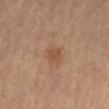• notes: total-body-photography surveillance lesion; no biopsy
• diameter: about 2.5 mm
• site: the abdomen
• tile lighting: cross-polarized illumination
• patient: male, aged 68 to 72
• image: ~15 mm tile from a whole-body skin photo